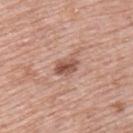Q: Was this lesion biopsied?
A: total-body-photography surveillance lesion; no biopsy
Q: Patient demographics?
A: male, aged around 55
Q: What is the imaging modality?
A: 15 mm crop, total-body photography
Q: What is the anatomic site?
A: the upper back
Q: How large is the lesion?
A: about 3 mm